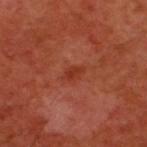The recorded lesion diameter is about 2.5 mm. This is a cross-polarized tile. Automated tile analysis of the lesion measured an area of roughly 3 mm² and two-axis asymmetry of about 0.25. A close-up tile cropped from a whole-body skin photograph, about 15 mm across. A male patient aged around 60. On the upper back.